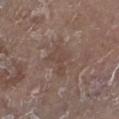Part of a total-body skin-imaging series; this lesion was reviewed on a skin check and was not flagged for biopsy. A male subject, aged 63 to 67. Captured under white-light illumination. The lesion is on the leg. About 3.5 mm across. A region of skin cropped from a whole-body photographic capture, roughly 15 mm wide.